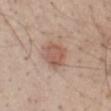Part of a total-body skin-imaging series; this lesion was reviewed on a skin check and was not flagged for biopsy. A male subject, approximately 60 years of age. A 15 mm crop from a total-body photograph taken for skin-cancer surveillance. Located on the abdomen.